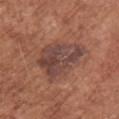anatomic site — the back | subject — female, aged around 75 | acquisition — ~15 mm tile from a whole-body skin photo | automated metrics — a footprint of about 22 mm², an eccentricity of roughly 0.7, and a shape-asymmetry score of about 0.35 (0 = symmetric); a lesion color around L≈44 a*≈20 b*≈23 in CIELAB, a lesion–skin lightness drop of about 9, and a lesion-to-skin contrast of about 8.5 (normalized; higher = more distinct); a border-irregularity index near 3.5/10 and a within-lesion color-variation index near 5/10; an automated nevus-likeness rating near 15 out of 100 and lesion-presence confidence of about 100/100.